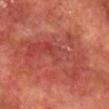biopsy status = total-body-photography surveillance lesion; no biopsy
acquisition = ~15 mm crop, total-body skin-cancer survey
automated lesion analysis = an area of roughly 32 mm² and two-axis asymmetry of about 0.6; a border-irregularity index near 9.5/10 and radial color variation of about 2.5
site = the chest
patient = male, aged 63 to 67